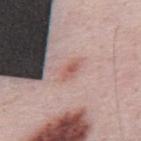Clinical impression: Recorded during total-body skin imaging; not selected for excision or biopsy. Clinical summary: An algorithmic analysis of the crop reported a lesion area of about 2.5 mm² and a symmetry-axis asymmetry near 0.3. The software also gave a nevus-likeness score of about 20/100 and a lesion-detection confidence of about 100/100. A roughly 15 mm field-of-view crop from a total-body skin photograph. From the upper back. The lesion's longest dimension is about 2.5 mm. A male subject about 50 years old. Imaged with white-light lighting.